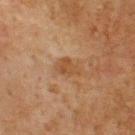workup = total-body-photography surveillance lesion; no biopsy | imaging modality = 15 mm crop, total-body photography | patient = male, in their mid-70s | diameter = ≈3 mm | body site = the upper back.